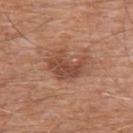<tbp_lesion>
  <biopsy_status>not biopsied; imaged during a skin examination</biopsy_status>
  <automated_metrics>
    <vs_skin_darker_L>10.0</vs_skin_darker_L>
    <vs_skin_contrast_norm>7.5</vs_skin_contrast_norm>
    <border_irregularity_0_10>5.0</border_irregularity_0_10>
    <peripheral_color_asymmetry>1.0</peripheral_color_asymmetry>
  </automated_metrics>
  <lighting>white-light</lighting>
  <lesion_size>
    <long_diameter_mm_approx>4.5</long_diameter_mm_approx>
  </lesion_size>
  <patient>
    <sex>male</sex>
    <age_approx>60</age_approx>
  </patient>
  <image>
    <source>total-body photography crop</source>
    <field_of_view_mm>15</field_of_view_mm>
  </image>
  <site>chest</site>
</tbp_lesion>